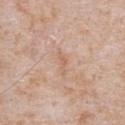Clinical impression: Part of a total-body skin-imaging series; this lesion was reviewed on a skin check and was not flagged for biopsy. Background: An algorithmic analysis of the crop reported border irregularity of about 5.5 on a 0–10 scale. About 3 mm across. Cropped from a total-body skin-imaging series; the visible field is about 15 mm. Imaged with white-light lighting. A male patient aged 63–67. On the abdomen.